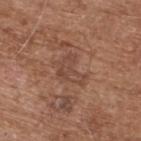image source: ~15 mm tile from a whole-body skin photo | anatomic site: the upper back | image-analysis metrics: an area of roughly 7.5 mm², a shape eccentricity near 0.7, and a shape-asymmetry score of about 0.55 (0 = symmetric); a border-irregularity index near 6/10 and a peripheral color-asymmetry measure near 1; an automated nevus-likeness rating near 0 out of 100 | illumination: white-light illumination | lesion diameter: ~4 mm (longest diameter) | subject: male, approximately 75 years of age.